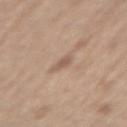Notes:
• follow-up: imaged on a skin check; not biopsied
• body site: the back
• lesion diameter: ≈2.5 mm
• image source: ~15 mm crop, total-body skin-cancer survey
• patient: male, roughly 70 years of age
• illumination: white-light